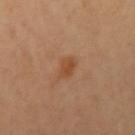Q: Was a biopsy performed?
A: total-body-photography surveillance lesion; no biopsy
Q: Who is the patient?
A: female, aged 38–42
Q: What is the lesion's diameter?
A: ~2.5 mm (longest diameter)
Q: What is the anatomic site?
A: the left arm
Q: Illumination type?
A: cross-polarized illumination
Q: What is the imaging modality?
A: 15 mm crop, total-body photography
Q: Automated lesion metrics?
A: a lesion area of about 3.5 mm², a shape eccentricity near 0.75, and a symmetry-axis asymmetry near 0.25